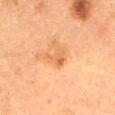Clinical impression:
The lesion was tiled from a total-body skin photograph and was not biopsied.
Clinical summary:
Approximately 2.5 mm at its widest. The patient is a female approximately 50 years of age. A lesion tile, about 15 mm wide, cut from a 3D total-body photograph. Located on the abdomen.